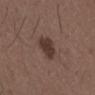Assessment: Captured during whole-body skin photography for melanoma surveillance; the lesion was not biopsied. Image and clinical context: Imaged with white-light lighting. Cropped from a whole-body photographic skin survey; the tile spans about 15 mm. A male subject, aged 48–52. The lesion is located on the front of the torso. Automated image analysis of the tile measured a lesion–skin lightness drop of about 10 and a normalized lesion–skin contrast near 9.5. The analysis additionally found a border-irregularity index near 2.5/10, a within-lesion color-variation index near 1.5/10, and radial color variation of about 0.5.